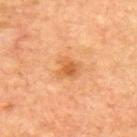Case summary:
– follow-up — imaged on a skin check; not biopsied